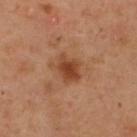<case>
<image>
  <source>total-body photography crop</source>
  <field_of_view_mm>15</field_of_view_mm>
</image>
<site>upper back</site>
<patient>
  <sex>male</sex>
  <age_approx>55</age_approx>
</patient>
</case>